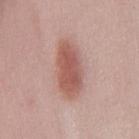Clinical impression: This lesion was catalogued during total-body skin photography and was not selected for biopsy. Context: The patient is a female about 40 years old. From the front of the torso. Cropped from a whole-body photographic skin survey; the tile spans about 15 mm. The recorded lesion diameter is about 6 mm. Captured under white-light illumination.